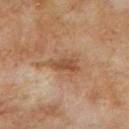Imaged during a routine full-body skin examination; the lesion was not biopsied and no histopathology is available. The recorded lesion diameter is about 5.5 mm. The lesion is located on the back. A male subject, aged around 70. A region of skin cropped from a whole-body photographic capture, roughly 15 mm wide. The lesion-visualizer software estimated a footprint of about 11 mm², a shape eccentricity near 0.8, and two-axis asymmetry of about 0.45. It also reported a mean CIELAB color near L≈51 a*≈19 b*≈33 and a normalized lesion–skin contrast near 6.5. This is a cross-polarized tile.